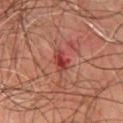  biopsy_status: not biopsied; imaged during a skin examination
  site: chest
  lighting: cross-polarized
  patient:
    sex: male
    age_approx: 70
  image:
    source: total-body photography crop
    field_of_view_mm: 15
  lesion_size:
    long_diameter_mm_approx: 3.0